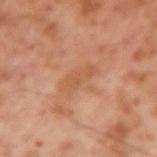Q: Was this lesion biopsied?
A: catalogued during a skin exam; not biopsied
Q: What kind of image is this?
A: 15 mm crop, total-body photography
Q: Where on the body is the lesion?
A: the left upper arm
Q: Who is the patient?
A: male, aged 53–57
Q: How large is the lesion?
A: ≈4.5 mm